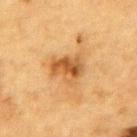Q: Is there a histopathology result?
A: total-body-photography surveillance lesion; no biopsy
Q: What are the patient's age and sex?
A: male, in their mid- to late 80s or older
Q: What kind of image is this?
A: ~15 mm tile from a whole-body skin photo
Q: Where on the body is the lesion?
A: the upper back
Q: What lighting was used for the tile?
A: cross-polarized illumination
Q: Automated lesion metrics?
A: a footprint of about 9.5 mm², an outline eccentricity of about 0.7 (0 = round, 1 = elongated), and two-axis asymmetry of about 0.35
Q: How large is the lesion?
A: ~4 mm (longest diameter)